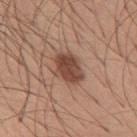No biopsy was performed on this lesion — it was imaged during a full skin examination and was not determined to be concerning.
From the back.
The tile uses white-light illumination.
The subject is a male in their 30s.
Approximately 4 mm at its widest.
A 15 mm crop from a total-body photograph taken for skin-cancer surveillance.